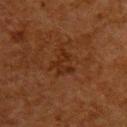workup: catalogued during a skin exam; not biopsied
imaging modality: ~15 mm tile from a whole-body skin photo
body site: the upper back
lighting: cross-polarized illumination
lesion diameter: about 3 mm
patient: female, about 50 years old